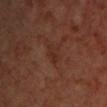Notes:
* workup · catalogued during a skin exam; not biopsied
* location · the chest
* TBP lesion metrics · a footprint of about 4 mm² and an outline eccentricity of about 0.85 (0 = round, 1 = elongated); an average lesion color of about L≈27 a*≈19 b*≈25 (CIELAB), roughly 5 lightness units darker than nearby skin, and a normalized border contrast of about 5.5
* acquisition · total-body-photography crop, ~15 mm field of view
* tile lighting · cross-polarized illumination
* patient · male, aged 68 to 72
* lesion diameter · about 3 mm